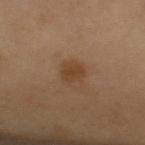follow-up — catalogued during a skin exam; not biopsied
lesion size — ~2.5 mm (longest diameter)
image — ~15 mm crop, total-body skin-cancer survey
image-analysis metrics — an area of roughly 4.5 mm², an outline eccentricity of about 0.7 (0 = round, 1 = elongated), and two-axis asymmetry of about 0.25; roughly 8 lightness units darker than nearby skin and a lesion-to-skin contrast of about 7 (normalized; higher = more distinct); a lesion-detection confidence of about 100/100
site — the right upper arm
patient — female, in their mid- to late 60s
tile lighting — cross-polarized illumination